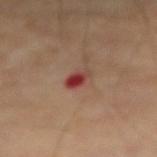No biopsy was performed on this lesion — it was imaged during a full skin examination and was not determined to be concerning.
The lesion is on the right forearm.
The lesion's longest dimension is about 2.5 mm.
The lesion-visualizer software estimated a lesion area of about 4 mm² and an outline eccentricity of about 0.75 (0 = round, 1 = elongated). It also reported a mean CIELAB color near L≈39 a*≈27 b*≈25, about 12 CIELAB-L* units darker than the surrounding skin, and a normalized border contrast of about 9.5. The software also gave border irregularity of about 1.5 on a 0–10 scale, a within-lesion color-variation index near 9.5/10, and radial color variation of about 3.5.
Captured under cross-polarized illumination.
A region of skin cropped from a whole-body photographic capture, roughly 15 mm wide.
A male patient, aged 68–72.